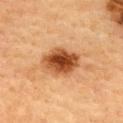| field | value |
|---|---|
| tile lighting | cross-polarized |
| patient | female, aged approximately 60 |
| lesion diameter | ≈5 mm |
| image | ~15 mm tile from a whole-body skin photo |
| anatomic site | the upper back |